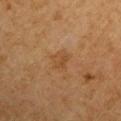{
  "biopsy_status": "not biopsied; imaged during a skin examination",
  "image": {
    "source": "total-body photography crop",
    "field_of_view_mm": 15
  },
  "lighting": "cross-polarized",
  "site": "left upper arm",
  "patient": {
    "sex": "male",
    "age_approx": 65
  }
}